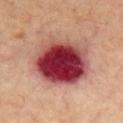Background: The lesion is located on the chest. A male patient aged around 70. A 15 mm crop from a total-body photograph taken for skin-cancer surveillance. Captured under cross-polarized illumination. Approximately 7.5 mm at its widest.